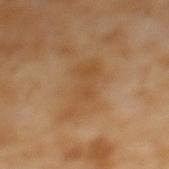{
  "biopsy_status": "not biopsied; imaged during a skin examination",
  "site": "mid back",
  "automated_metrics": {
    "vs_skin_darker_L": 7.0,
    "nevus_likeness_0_100": 0,
    "lesion_detection_confidence_0_100": 100
  },
  "lesion_size": {
    "long_diameter_mm_approx": 6.0
  },
  "patient": {
    "sex": "female",
    "age_approx": 55
  },
  "lighting": "cross-polarized",
  "image": {
    "source": "total-body photography crop",
    "field_of_view_mm": 15
  }
}